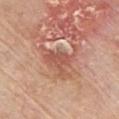| field | value |
|---|---|
| workup | no biopsy performed (imaged during a skin exam) |
| lighting | white-light illumination |
| subject | male, approximately 80 years of age |
| site | the front of the torso |
| size | ~5 mm (longest diameter) |
| image source | total-body-photography crop, ~15 mm field of view |